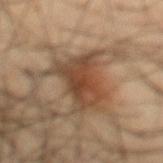Case summary:
* notes — catalogued during a skin exam; not biopsied
* body site — the leg
* image — ~15 mm tile from a whole-body skin photo
* image-analysis metrics — a footprint of about 18 mm², a shape eccentricity near 0.4, and a shape-asymmetry score of about 0.35 (0 = symmetric); an average lesion color of about L≈43 a*≈18 b*≈30 (CIELAB) and a normalized border contrast of about 8.5
* diameter — about 6.5 mm
* tile lighting — cross-polarized illumination
* patient — male, aged around 45